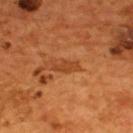notes — no biopsy performed (imaged during a skin exam)
patient — male, approximately 55 years of age
imaging modality — ~15 mm crop, total-body skin-cancer survey
TBP lesion metrics — a footprint of about 3.5 mm², an outline eccentricity of about 0.9 (0 = round, 1 = elongated), and a shape-asymmetry score of about 0.35 (0 = symmetric); a mean CIELAB color near L≈41 a*≈26 b*≈38, roughly 8 lightness units darker than nearby skin, and a normalized lesion–skin contrast near 6; a nevus-likeness score of about 10/100 and a lesion-detection confidence of about 90/100
anatomic site — the upper back
illumination — cross-polarized
size — ≈3.5 mm